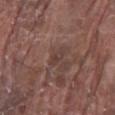– workup: no biopsy performed (imaged during a skin exam)
– image source: ~15 mm tile from a whole-body skin photo
– anatomic site: the lower back
– tile lighting: white-light illumination
– subject: male, aged around 80
– image-analysis metrics: an area of roughly 3 mm², a shape eccentricity near 0.9, and two-axis asymmetry of about 0.3; a lesion color around L≈39 a*≈17 b*≈21 in CIELAB and a lesion-to-skin contrast of about 5.5 (normalized; higher = more distinct); a border-irregularity index near 3/10 and radial color variation of about 0